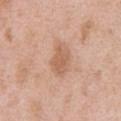Q: Was this lesion biopsied?
A: total-body-photography surveillance lesion; no biopsy
Q: Lesion location?
A: the chest
Q: What are the patient's age and sex?
A: male, aged 48 to 52
Q: What kind of image is this?
A: total-body-photography crop, ~15 mm field of view
Q: How large is the lesion?
A: ~3.5 mm (longest diameter)